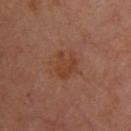Approximately 3 mm at its widest. The lesion is on the upper back. Automated image analysis of the tile measured an area of roughly 6.5 mm² and a symmetry-axis asymmetry near 0.35. The software also gave border irregularity of about 5 on a 0–10 scale, internal color variation of about 2 on a 0–10 scale, and a peripheral color-asymmetry measure near 0.5. The tile uses cross-polarized illumination. Cropped from a whole-body photographic skin survey; the tile spans about 15 mm. The subject is a female aged 43 to 47.